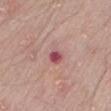Assessment:
The lesion was tiled from a total-body skin photograph and was not biopsied.